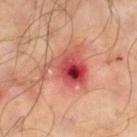| key | value |
|---|---|
| notes | imaged on a skin check; not biopsied |
| diameter | ≈6 mm |
| image | ~15 mm crop, total-body skin-cancer survey |
| subject | male, approximately 65 years of age |
| automated metrics | an area of roughly 16 mm², an outline eccentricity of about 0.55 (0 = round, 1 = elongated), and a symmetry-axis asymmetry near 0.5; a border-irregularity index near 6/10 and a peripheral color-asymmetry measure near 5.5 |
| anatomic site | the right lower leg |
| tile lighting | cross-polarized |